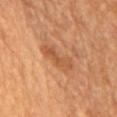Part of a total-body skin-imaging series; this lesion was reviewed on a skin check and was not flagged for biopsy.
A 15 mm close-up extracted from a 3D total-body photography capture.
The lesion-visualizer software estimated a shape eccentricity near 0.95 and a shape-asymmetry score of about 0.3 (0 = symmetric). And it measured a classifier nevus-likeness of about 45/100 and lesion-presence confidence of about 95/100.
Longest diameter approximately 5 mm.
Imaged with cross-polarized lighting.
The subject is a female in their 70s.
Located on the front of the torso.